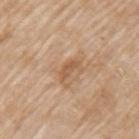<record>
  <biopsy_status>not biopsied; imaged during a skin examination</biopsy_status>
  <lighting>white-light</lighting>
  <automated_metrics>
    <eccentricity>0.9</eccentricity>
    <vs_skin_darker_L>9.0</vs_skin_darker_L>
    <vs_skin_contrast_norm>6.0</vs_skin_contrast_norm>
    <border_irregularity_0_10>3.0</border_irregularity_0_10>
    <color_variation_0_10>0.0</color_variation_0_10>
    <peripheral_color_asymmetry>0.0</peripheral_color_asymmetry>
    <nevus_likeness_0_100>0</nevus_likeness_0_100>
    <lesion_detection_confidence_0_100>100</lesion_detection_confidence_0_100>
  </automated_metrics>
  <image>
    <source>total-body photography crop</source>
    <field_of_view_mm>15</field_of_view_mm>
  </image>
  <patient>
    <sex>female</sex>
    <age_approx>75</age_approx>
  </patient>
  <lesion_size>
    <long_diameter_mm_approx>2.5</long_diameter_mm_approx>
  </lesion_size>
  <site>left upper arm</site>
</record>